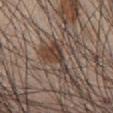| key | value |
|---|---|
| workup | catalogued during a skin exam; not biopsied |
| image source | 15 mm crop, total-body photography |
| automated metrics | a normalized lesion–skin contrast near 9; a border-irregularity rating of about 7.5/10; a nevus-likeness score of about 85/100 and a lesion-detection confidence of about 90/100 |
| lighting | white-light |
| subject | male, aged around 65 |
| size | ~4.5 mm (longest diameter) |
| anatomic site | the abdomen |